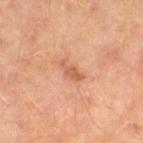Assessment: The lesion was tiled from a total-body skin photograph and was not biopsied. Image and clinical context: Cropped from a total-body skin-imaging series; the visible field is about 15 mm. This is a cross-polarized tile. The subject is a male aged around 60. From the leg. About 3 mm across.